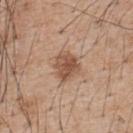The lesion was tiled from a total-body skin photograph and was not biopsied.
The recorded lesion diameter is about 3.5 mm.
The lesion is on the upper back.
A male patient, aged around 55.
A region of skin cropped from a whole-body photographic capture, roughly 15 mm wide.
The tile uses white-light illumination.